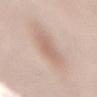Notes:
* biopsy status · imaged on a skin check; not biopsied
* site · the mid back
* patient · female, aged around 50
* lighting · white-light
* size · ≈4 mm
* imaging modality · total-body-photography crop, ~15 mm field of view